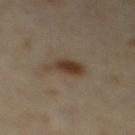No biopsy was performed on this lesion — it was imaged during a full skin examination and was not determined to be concerning.